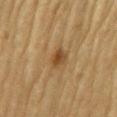biopsy status = total-body-photography surveillance lesion; no biopsy | illumination = cross-polarized | patient = male, aged around 85 or older | acquisition = ~15 mm crop, total-body skin-cancer survey | body site = the arm | size = ≈3 mm.